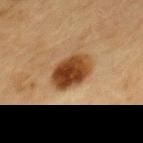follow-up — catalogued during a skin exam; not biopsied | patient — female, aged approximately 60 | site — the upper back | imaging modality — ~15 mm crop, total-body skin-cancer survey.